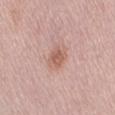biopsy status = total-body-photography surveillance lesion; no biopsy | lesion diameter = about 2.5 mm | imaging modality = 15 mm crop, total-body photography | automated lesion analysis = an area of roughly 5 mm² and two-axis asymmetry of about 0.2; an average lesion color of about L≈59 a*≈22 b*≈27 (CIELAB), about 9 CIELAB-L* units darker than the surrounding skin, and a normalized lesion–skin contrast near 6.5; a lesion-detection confidence of about 100/100 | body site = the right thigh | patient = female, about 50 years old | illumination = white-light.